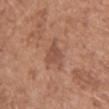Context:
The lesion is on the front of the torso. Longest diameter approximately 4 mm. A female subject, about 75 years old. This is a white-light tile. A region of skin cropped from a whole-body photographic capture, roughly 15 mm wide.